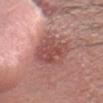The lesion was photographed on a routine skin check and not biopsied; there is no pathology result.
A close-up tile cropped from a whole-body skin photograph, about 15 mm across.
The subject is a male roughly 30 years of age.
Measured at roughly 6 mm in maximum diameter.